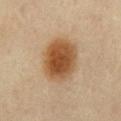Clinical impression: The lesion was photographed on a routine skin check and not biopsied; there is no pathology result. Context: On the abdomen. Captured under cross-polarized illumination. A female patient, aged around 65. Measured at roughly 5.5 mm in maximum diameter. Cropped from a total-body skin-imaging series; the visible field is about 15 mm.